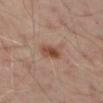notes: total-body-photography surveillance lesion; no biopsy | image source: ~15 mm tile from a whole-body skin photo | site: the abdomen | lesion size: ~3 mm (longest diameter) | TBP lesion metrics: a border-irregularity index near 2.5/10, a color-variation rating of about 3.5/10, and a peripheral color-asymmetry measure near 1; an automated nevus-likeness rating near 95 out of 100 and a lesion-detection confidence of about 100/100 | patient: male, aged approximately 50 | illumination: cross-polarized.